From the arm. A female patient aged 58–62. A close-up tile cropped from a whole-body skin photograph, about 15 mm across: 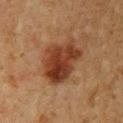<case>
  <lighting>cross-polarized</lighting>
  <lesion_size>
    <long_diameter_mm_approx>6.0</long_diameter_mm_approx>
  </lesion_size>
  <diagnosis>
    <histopathology>dysplastic (Clark) nevus</histopathology>
    <malignancy>benign</malignancy>
    <taxonomic_path>Benign; Benign melanocytic proliferations; Nevus; Nevus, Atypical, Dysplastic, or Clark</taxonomic_path>
  </diagnosis>
</case>